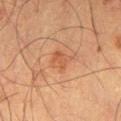The lesion was photographed on a routine skin check and not biopsied; there is no pathology result.
The lesion is located on the leg.
Cropped from a total-body skin-imaging series; the visible field is about 15 mm.
About 3 mm across.
The subject is a male in their mid- to late 60s.